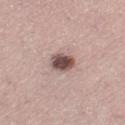Q: Was a biopsy performed?
A: imaged on a skin check; not biopsied
Q: Automated lesion metrics?
A: an outline eccentricity of about 0.55 (0 = round, 1 = elongated) and a shape-asymmetry score of about 0.15 (0 = symmetric); a mean CIELAB color near L≈51 a*≈17 b*≈20, a lesion–skin lightness drop of about 17, and a normalized lesion–skin contrast near 11; a border-irregularity index near 1.5/10, a color-variation rating of about 4.5/10, and radial color variation of about 1; a classifier nevus-likeness of about 75/100 and lesion-presence confidence of about 100/100
Q: Patient demographics?
A: female, aged 38 to 42
Q: What is the anatomic site?
A: the left thigh
Q: How was this image acquired?
A: 15 mm crop, total-body photography
Q: How was the tile lit?
A: white-light illumination